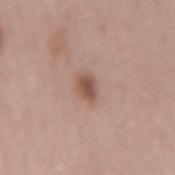Imaged during a routine full-body skin examination; the lesion was not biopsied and no histopathology is available.
From the mid back.
A female subject, about 65 years old.
A roughly 15 mm field-of-view crop from a total-body skin photograph.
The recorded lesion diameter is about 2.5 mm.